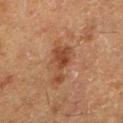<record>
<biopsy_status>not biopsied; imaged during a skin examination</biopsy_status>
<site>left lower leg</site>
<patient>
  <sex>female</sex>
  <age_approx>50</age_approx>
</patient>
<lesion_size>
  <long_diameter_mm_approx>4.5</long_diameter_mm_approx>
</lesion_size>
<lighting>cross-polarized</lighting>
<automated_metrics>
  <area_mm2_approx>8.0</area_mm2_approx>
  <shape_asymmetry>0.55</shape_asymmetry>
  <vs_skin_darker_L>8.0</vs_skin_darker_L>
</automated_metrics>
<image>
  <source>total-body photography crop</source>
  <field_of_view_mm>15</field_of_view_mm>
</image>
</record>